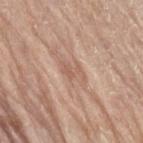Assessment: Recorded during total-body skin imaging; not selected for excision or biopsy. Acquisition and patient details: A male patient, about 80 years old. The total-body-photography lesion software estimated a lesion color around L≈58 a*≈19 b*≈28 in CIELAB, roughly 8 lightness units darker than nearby skin, and a normalized border contrast of about 5. And it measured a within-lesion color-variation index near 1/10 and peripheral color asymmetry of about 0.5. It also reported a detector confidence of about 75 out of 100 that the crop contains a lesion. The tile uses white-light illumination. Located on the left upper arm. Cropped from a whole-body photographic skin survey; the tile spans about 15 mm. Measured at roughly 3 mm in maximum diameter.